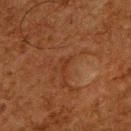workup: catalogued during a skin exam; not biopsied | body site: the upper back | tile lighting: cross-polarized illumination | size: ~2.5 mm (longest diameter) | imaging modality: ~15 mm tile from a whole-body skin photo | patient: male, aged 63 to 67 | image-analysis metrics: a mean CIELAB color near L≈29 a*≈21 b*≈30, a lesion–skin lightness drop of about 4, and a lesion-to-skin contrast of about 5 (normalized; higher = more distinct); an automated nevus-likeness rating near 0 out of 100 and lesion-presence confidence of about 70/100.